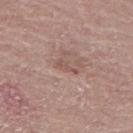Q: Was a biopsy performed?
A: no biopsy performed (imaged during a skin exam)
Q: What is the lesion's diameter?
A: ~2.5 mm (longest diameter)
Q: Lesion location?
A: the left thigh
Q: What did automated image analysis measure?
A: a border-irregularity index near 5.5/10, internal color variation of about 0 on a 0–10 scale, and a peripheral color-asymmetry measure near 0; a nevus-likeness score of about 0/100 and a detector confidence of about 100 out of 100 that the crop contains a lesion
Q: How was this image acquired?
A: 15 mm crop, total-body photography
Q: What are the patient's age and sex?
A: female, aged around 65